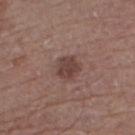No biopsy was performed on this lesion — it was imaged during a full skin examination and was not determined to be concerning. A male patient aged approximately 65. From the left lower leg. Cropped from a whole-body photographic skin survey; the tile spans about 15 mm. This is a white-light tile.